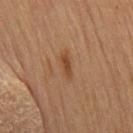The lesion was photographed on a routine skin check and not biopsied; there is no pathology result. The patient is a female aged approximately 80. An algorithmic analysis of the crop reported a footprint of about 2.5 mm², an outline eccentricity of about 0.95 (0 = round, 1 = elongated), and a symmetry-axis asymmetry near 0.25. It also reported border irregularity of about 3 on a 0–10 scale, a color-variation rating of about 0/10, and radial color variation of about 0. The analysis additionally found a nevus-likeness score of about 75/100 and a lesion-detection confidence of about 100/100. A region of skin cropped from a whole-body photographic capture, roughly 15 mm wide. Imaged with cross-polarized lighting. The lesion is located on the leg.